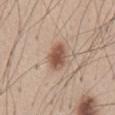notes=no biopsy performed (imaged during a skin exam)
image source=total-body-photography crop, ~15 mm field of view
lesion size=~3.5 mm (longest diameter)
tile lighting=white-light
patient=male, approximately 45 years of age
anatomic site=the abdomen